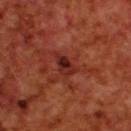biopsy status = imaged on a skin check; not biopsied
lesion size = ~3 mm (longest diameter)
imaging modality = ~15 mm crop, total-body skin-cancer survey
anatomic site = the upper back
subject = male, aged approximately 70